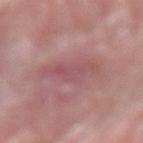Findings:
• notes · total-body-photography surveillance lesion; no biopsy
• acquisition · 15 mm crop, total-body photography
• patient · male, about 75 years old
• lesion size · ≈5.5 mm
• tile lighting · white-light
• body site · the right forearm